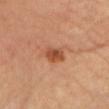Findings:
– biopsy status · imaged on a skin check; not biopsied
– image · total-body-photography crop, ~15 mm field of view
– subject · female, roughly 60 years of age
– location · the chest